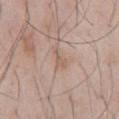The lesion was tiled from a total-body skin photograph and was not biopsied. The recorded lesion diameter is about 2.5 mm. The tile uses white-light illumination. Automated image analysis of the tile measured an eccentricity of roughly 0.9 and a shape-asymmetry score of about 0.5 (0 = symmetric). And it measured a mean CIELAB color near L≈59 a*≈17 b*≈26, roughly 7 lightness units darker than nearby skin, and a lesion-to-skin contrast of about 5 (normalized; higher = more distinct). And it measured a border-irregularity rating of about 5/10, a within-lesion color-variation index near 0.5/10, and peripheral color asymmetry of about 0. It also reported an automated nevus-likeness rating near 0 out of 100 and a lesion-detection confidence of about 80/100. A male subject, in their mid-50s. The lesion is on the abdomen. Cropped from a total-body skin-imaging series; the visible field is about 15 mm.